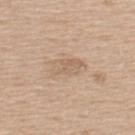Q: Was a biopsy performed?
A: no biopsy performed (imaged during a skin exam)
Q: What lighting was used for the tile?
A: white-light
Q: Lesion size?
A: ~4 mm (longest diameter)
Q: What is the anatomic site?
A: the upper back
Q: What kind of image is this?
A: ~15 mm crop, total-body skin-cancer survey
Q: Patient demographics?
A: male, approximately 60 years of age